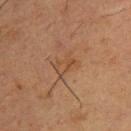Captured during whole-body skin photography for melanoma surveillance; the lesion was not biopsied. The recorded lesion diameter is about 3 mm. A lesion tile, about 15 mm wide, cut from a 3D total-body photograph. On the chest. A male patient about 55 years old. Captured under cross-polarized illumination.